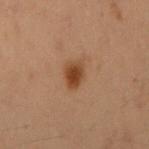Part of a total-body skin-imaging series; this lesion was reviewed on a skin check and was not flagged for biopsy. The tile uses cross-polarized illumination. The patient is a female approximately 40 years of age. The lesion-visualizer software estimated a shape eccentricity near 0.7 and a symmetry-axis asymmetry near 0.15. And it measured an average lesion color of about L≈35 a*≈18 b*≈29 (CIELAB) and a normalized lesion–skin contrast near 9.5. The analysis additionally found an automated nevus-likeness rating near 100 out of 100 and a lesion-detection confidence of about 100/100. Cropped from a total-body skin-imaging series; the visible field is about 15 mm. Located on the arm.